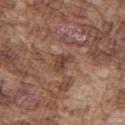The lesion was photographed on a routine skin check and not biopsied; there is no pathology result. The lesion is on the right upper arm. The lesion's longest dimension is about 3 mm. Imaged with white-light lighting. A lesion tile, about 15 mm wide, cut from a 3D total-body photograph. The patient is a male about 75 years old. The total-body-photography lesion software estimated a lesion color around L≈43 a*≈19 b*≈26 in CIELAB, roughly 9 lightness units darker than nearby skin, and a normalized border contrast of about 7.